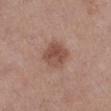A close-up tile cropped from a whole-body skin photograph, about 15 mm across. A female subject, aged 43 to 47. Located on the left lower leg.